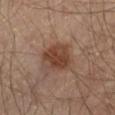Findings:
– notes: total-body-photography surveillance lesion; no biopsy
– automated lesion analysis: a footprint of about 10 mm², an eccentricity of roughly 0.7, and two-axis asymmetry of about 0.25; a lesion color around L≈40 a*≈20 b*≈27 in CIELAB, a lesion–skin lightness drop of about 10, and a normalized lesion–skin contrast near 9
– acquisition: ~15 mm tile from a whole-body skin photo
– illumination: cross-polarized illumination
– patient: male, in their mid-60s
– location: the leg